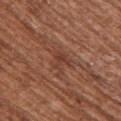{"biopsy_status": "not biopsied; imaged during a skin examination", "image": {"source": "total-body photography crop", "field_of_view_mm": 15}, "automated_metrics": {"shape_asymmetry": 0.55, "cielab_L": 39, "cielab_a": 24, "cielab_b": 29, "vs_skin_darker_L": 7.0, "vs_skin_contrast_norm": 6.0, "border_irregularity_0_10": 6.5, "color_variation_0_10": 0.5, "peripheral_color_asymmetry": 0.0, "nevus_likeness_0_100": 0, "lesion_detection_confidence_0_100": 95}, "patient": {"sex": "female", "age_approx": 80}, "lighting": "white-light", "lesion_size": {"long_diameter_mm_approx": 2.5}, "site": "right upper arm"}